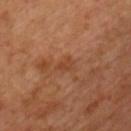workup: no biopsy performed (imaged during a skin exam) | diameter: ~2.5 mm (longest diameter) | imaging modality: 15 mm crop, total-body photography | subject: male, roughly 50 years of age | body site: the chest | illumination: cross-polarized illumination.